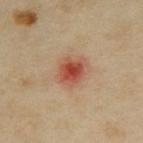biopsy status — no biopsy performed (imaged during a skin exam) | size — ≈3.5 mm | patient — female, in their mid-30s | tile lighting — cross-polarized illumination | body site — the back | imaging modality — ~15 mm crop, total-body skin-cancer survey.